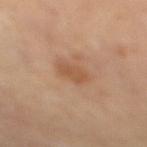workup — imaged on a skin check; not biopsied
patient — female, aged 48–52
acquisition — total-body-photography crop, ~15 mm field of view
lighting — cross-polarized
anatomic site — the right thigh
size — about 2.5 mm